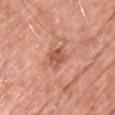Recorded during total-body skin imaging; not selected for excision or biopsy. The subject is a male aged approximately 60. Located on the chest. The lesion's longest dimension is about 3 mm. A 15 mm close-up tile from a total-body photography series done for melanoma screening. Automated image analysis of the tile measured a border-irregularity index near 2.5/10, internal color variation of about 3 on a 0–10 scale, and peripheral color asymmetry of about 1. It also reported a classifier nevus-likeness of about 0/100. Imaged with white-light lighting.